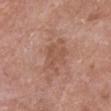Part of a total-body skin-imaging series; this lesion was reviewed on a skin check and was not flagged for biopsy.
On the front of the torso.
Automated image analysis of the tile measured a mean CIELAB color near L≈52 a*≈22 b*≈28 and roughly 7 lightness units darker than nearby skin. It also reported internal color variation of about 1.5 on a 0–10 scale and radial color variation of about 0.5. It also reported a classifier nevus-likeness of about 0/100.
A region of skin cropped from a whole-body photographic capture, roughly 15 mm wide.
Approximately 3.5 mm at its widest.
A female patient, in their 70s.
Captured under white-light illumination.